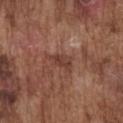The lesion was tiled from a total-body skin photograph and was not biopsied. The lesion's longest dimension is about 3.5 mm. A 15 mm close-up extracted from a 3D total-body photography capture. The lesion-visualizer software estimated a lesion color around L≈40 a*≈22 b*≈25 in CIELAB, a lesion–skin lightness drop of about 8, and a normalized border contrast of about 6.5. And it measured border irregularity of about 4 on a 0–10 scale, a color-variation rating of about 2.5/10, and radial color variation of about 1. The software also gave a classifier nevus-likeness of about 0/100 and a detector confidence of about 100 out of 100 that the crop contains a lesion. This is a white-light tile. The lesion is on the chest. A male subject aged approximately 75.